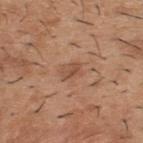<case>
<lighting>white-light</lighting>
<site>upper back</site>
<lesion_size>
  <long_diameter_mm_approx>2.5</long_diameter_mm_approx>
</lesion_size>
<image>
  <source>total-body photography crop</source>
  <field_of_view_mm>15</field_of_view_mm>
</image>
<patient>
  <sex>male</sex>
  <age_approx>40</age_approx>
</patient>
</case>